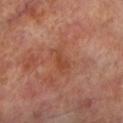Part of a total-body skin-imaging series; this lesion was reviewed on a skin check and was not flagged for biopsy.
Captured under cross-polarized illumination.
About 2.5 mm across.
Automated image analysis of the tile measured a border-irregularity index near 4/10, a color-variation rating of about 0.5/10, and a peripheral color-asymmetry measure near 0. The analysis additionally found a nevus-likeness score of about 0/100 and a lesion-detection confidence of about 100/100.
A male subject, approximately 70 years of age.
From the left lower leg.
A close-up tile cropped from a whole-body skin photograph, about 15 mm across.